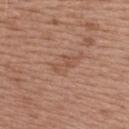Part of a total-body skin-imaging series; this lesion was reviewed on a skin check and was not flagged for biopsy.
The subject is a female about 40 years old.
The recorded lesion diameter is about 3 mm.
This is a white-light tile.
This image is a 15 mm lesion crop taken from a total-body photograph.
From the upper back.